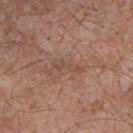Part of a total-body skin-imaging series; this lesion was reviewed on a skin check and was not flagged for biopsy. The lesion is located on the front of the torso. Cropped from a whole-body photographic skin survey; the tile spans about 15 mm. The tile uses white-light illumination. A male subject aged around 70.